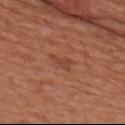Clinical impression: The lesion was tiled from a total-body skin photograph and was not biopsied. Context: The recorded lesion diameter is about 2.5 mm. From the back. A male patient, roughly 65 years of age. Captured under white-light illumination. A 15 mm close-up extracted from a 3D total-body photography capture. The lesion-visualizer software estimated border irregularity of about 5 on a 0–10 scale and a within-lesion color-variation index near 0/10.